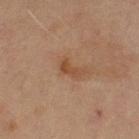  biopsy_status: not biopsied; imaged during a skin examination